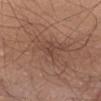<record>
<biopsy_status>not biopsied; imaged during a skin examination</biopsy_status>
<image>
  <source>total-body photography crop</source>
  <field_of_view_mm>15</field_of_view_mm>
</image>
<patient>
  <sex>male</sex>
  <age_approx>55</age_approx>
</patient>
<lesion_size>
  <long_diameter_mm_approx>2.5</long_diameter_mm_approx>
</lesion_size>
<lighting>white-light</lighting>
<site>chest</site>
</record>